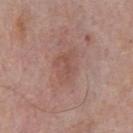Assessment:
The lesion was tiled from a total-body skin photograph and was not biopsied.
Acquisition and patient details:
Longest diameter approximately 4 mm. The patient is a male in their mid-70s. A roughly 15 mm field-of-view crop from a total-body skin photograph. The lesion is located on the chest. This is a white-light tile.